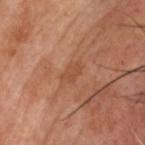{
  "biopsy_status": "not biopsied; imaged during a skin examination",
  "lesion_size": {
    "long_diameter_mm_approx": 2.5
  },
  "automated_metrics": {
    "eccentricity": 0.8,
    "shape_asymmetry": 0.3,
    "vs_skin_darker_L": 6.0,
    "vs_skin_contrast_norm": 5.0
  },
  "lighting": "cross-polarized",
  "site": "head or neck",
  "image": {
    "source": "total-body photography crop",
    "field_of_view_mm": 15
  },
  "patient": {
    "sex": "male",
    "age_approx": 70
  }
}